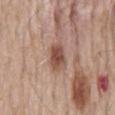| field | value |
|---|---|
| follow-up | no biopsy performed (imaged during a skin exam) |
| acquisition | total-body-photography crop, ~15 mm field of view |
| subject | male, about 55 years old |
| lesion size | ≈3 mm |
| body site | the back |